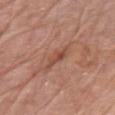| key | value |
|---|---|
| biopsy status | imaged on a skin check; not biopsied |
| acquisition | 15 mm crop, total-body photography |
| illumination | white-light illumination |
| subject | male, aged 78–82 |
| automated lesion analysis | a footprint of about 5.5 mm², an eccentricity of roughly 0.95, and a shape-asymmetry score of about 0.35 (0 = symmetric); an average lesion color of about L≈50 a*≈23 b*≈29 (CIELAB) and a normalized lesion–skin contrast near 6; a border-irregularity rating of about 4.5/10, internal color variation of about 3.5 on a 0–10 scale, and a peripheral color-asymmetry measure near 1.5; an automated nevus-likeness rating near 0 out of 100 and a lesion-detection confidence of about 80/100 |
| body site | the front of the torso |
| diameter | ~4.5 mm (longest diameter) |